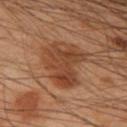Q: Was this lesion biopsied?
A: no biopsy performed (imaged during a skin exam)
Q: What is the lesion's diameter?
A: ~7 mm (longest diameter)
Q: Lesion location?
A: the left forearm
Q: What kind of image is this?
A: total-body-photography crop, ~15 mm field of view
Q: How was the tile lit?
A: cross-polarized
Q: Patient demographics?
A: male, aged 48–52